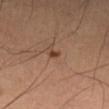Q: Is there a histopathology result?
A: imaged on a skin check; not biopsied
Q: Patient demographics?
A: male, approximately 65 years of age
Q: How was this image acquired?
A: ~15 mm crop, total-body skin-cancer survey
Q: Lesion size?
A: ≈1.5 mm
Q: Lesion location?
A: the leg
Q: What lighting was used for the tile?
A: cross-polarized illumination